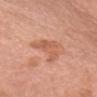Assessment:
Part of a total-body skin-imaging series; this lesion was reviewed on a skin check and was not flagged for biopsy.
Background:
Captured under white-light illumination. Automated image analysis of the tile measured a footprint of about 8 mm², a shape eccentricity near 0.75, and a symmetry-axis asymmetry near 0.55. The analysis additionally found a lesion color around L≈59 a*≈26 b*≈34 in CIELAB and roughly 8 lightness units darker than nearby skin. And it measured a border-irregularity index near 6.5/10, a within-lesion color-variation index near 2/10, and radial color variation of about 0.5. The patient is a female roughly 65 years of age. Located on the head or neck. This image is a 15 mm lesion crop taken from a total-body photograph.